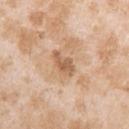{"biopsy_status": "not biopsied; imaged during a skin examination", "patient": {"sex": "female", "age_approx": 25}, "lesion_size": {"long_diameter_mm_approx": 3.5}, "site": "right upper arm", "image": {"source": "total-body photography crop", "field_of_view_mm": 15}, "automated_metrics": {"cielab_L": 60, "cielab_a": 20, "cielab_b": 34, "vs_skin_contrast_norm": 7.0, "nevus_likeness_0_100": 0, "lesion_detection_confidence_0_100": 100}, "lighting": "white-light"}